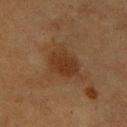workup: imaged on a skin check; not biopsied
acquisition: 15 mm crop, total-body photography
image-analysis metrics: an outline eccentricity of about 0.65 (0 = round, 1 = elongated); a lesion color around L≈27 a*≈16 b*≈26 in CIELAB, roughly 7 lightness units darker than nearby skin, and a normalized border contrast of about 7.5; a border-irregularity index near 2/10; a classifier nevus-likeness of about 25/100 and a detector confidence of about 100 out of 100 that the crop contains a lesion
lesion size: ≈4 mm
subject: male, about 75 years old
tile lighting: cross-polarized
location: the front of the torso